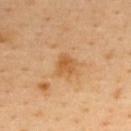Q: Was this lesion biopsied?
A: no biopsy performed (imaged during a skin exam)
Q: Lesion size?
A: ~2.5 mm (longest diameter)
Q: How was the tile lit?
A: cross-polarized
Q: Where on the body is the lesion?
A: the back
Q: What did automated image analysis measure?
A: a classifier nevus-likeness of about 5/100 and a detector confidence of about 100 out of 100 that the crop contains a lesion
Q: How was this image acquired?
A: ~15 mm crop, total-body skin-cancer survey
Q: Patient demographics?
A: male, roughly 40 years of age